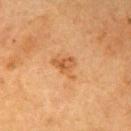Recorded during total-body skin imaging; not selected for excision or biopsy.
A roughly 15 mm field-of-view crop from a total-body skin photograph.
From the right upper arm.
A female patient, aged around 55.